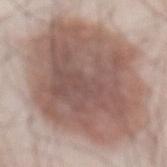Captured during whole-body skin photography for melanoma surveillance; the lesion was not biopsied. The lesion is located on the front of the torso. A close-up tile cropped from a whole-body skin photograph, about 15 mm across. The subject is a male aged around 55.